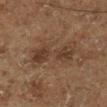biopsy status=no biopsy performed (imaged during a skin exam) | lighting=cross-polarized | subject=male, aged 73 to 77 | location=the leg | diameter=≈6 mm | image source=total-body-photography crop, ~15 mm field of view.